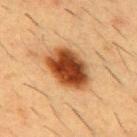Impression: Captured during whole-body skin photography for melanoma surveillance; the lesion was not biopsied. Image and clinical context: The patient is a male aged around 55. Measured at roughly 6 mm in maximum diameter. This is a cross-polarized tile. The lesion is on the chest. A lesion tile, about 15 mm wide, cut from a 3D total-body photograph.